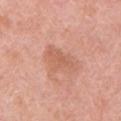Case summary:
* notes — total-body-photography surveillance lesion; no biopsy
* lesion size — ~4.5 mm (longest diameter)
* imaging modality — ~15 mm crop, total-body skin-cancer survey
* site — the left upper arm
* tile lighting — white-light
* subject — female, about 60 years old